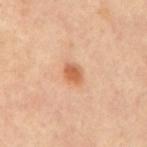Clinical summary: Longest diameter approximately 2.5 mm. This is a cross-polarized tile. The lesion is on the mid back. A male subject aged 53–57. A 15 mm close-up extracted from a 3D total-body photography capture.